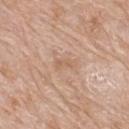Captured during whole-body skin photography for melanoma surveillance; the lesion was not biopsied. On the mid back. The total-body-photography lesion software estimated an area of roughly 2.5 mm² and a symmetry-axis asymmetry near 0.25. It also reported roughly 6 lightness units darker than nearby skin and a normalized border contrast of about 4.5. It also reported a border-irregularity rating of about 3/10 and peripheral color asymmetry of about 0. And it measured a detector confidence of about 100 out of 100 that the crop contains a lesion. Imaged with white-light lighting. A close-up tile cropped from a whole-body skin photograph, about 15 mm across. About 2.5 mm across. The patient is a male aged around 65.